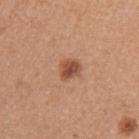• notes · catalogued during a skin exam; not biopsied
• imaging modality · ~15 mm tile from a whole-body skin photo
• patient · female, aged 33–37
• tile lighting · white-light illumination
• diameter · about 2.5 mm
• location · the right upper arm